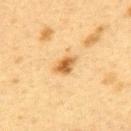The total-body-photography lesion software estimated a border-irregularity rating of about 2.5/10, a within-lesion color-variation index near 4.5/10, and peripheral color asymmetry of about 1.5. The software also gave an automated nevus-likeness rating near 80 out of 100 and a detector confidence of about 100 out of 100 that the crop contains a lesion.
The lesion is located on the upper back.
A female patient about 40 years old.
Measured at roughly 3 mm in maximum diameter.
Cropped from a whole-body photographic skin survey; the tile spans about 15 mm.
Imaged with cross-polarized lighting.